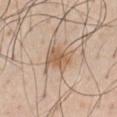follow-up=imaged on a skin check; not biopsied
automated lesion analysis=border irregularity of about 3 on a 0–10 scale, a within-lesion color-variation index near 3/10, and peripheral color asymmetry of about 1
location=the chest
acquisition=total-body-photography crop, ~15 mm field of view
subject=male, aged approximately 60